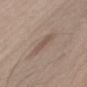Imaged during a routine full-body skin examination; the lesion was not biopsied and no histopathology is available.
The lesion-visualizer software estimated an area of roughly 4 mm². And it measured an average lesion color of about L≈52 a*≈15 b*≈23 (CIELAB) and a lesion-to-skin contrast of about 6 (normalized; higher = more distinct). It also reported a border-irregularity index near 4.5/10, a color-variation rating of about 0.5/10, and radial color variation of about 0. And it measured a nevus-likeness score of about 5/100 and a detector confidence of about 80 out of 100 that the crop contains a lesion.
A male patient aged approximately 40.
A roughly 15 mm field-of-view crop from a total-body skin photograph.
Captured under white-light illumination.
The recorded lesion diameter is about 3.5 mm.
The lesion is located on the mid back.